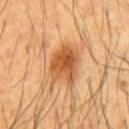<tbp_lesion>
  <biopsy_status>not biopsied; imaged during a skin examination</biopsy_status>
  <lesion_size>
    <long_diameter_mm_approx>5.0</long_diameter_mm_approx>
  </lesion_size>
  <site>front of the torso</site>
  <image>
    <source>total-body photography crop</source>
    <field_of_view_mm>15</field_of_view_mm>
  </image>
  <patient>
    <sex>male</sex>
    <age_approx>45</age_approx>
  </patient>
</tbp_lesion>